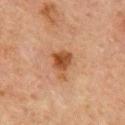| key | value |
|---|---|
| workup | no biopsy performed (imaged during a skin exam) |
| body site | the upper back |
| patient | male, aged around 65 |
| acquisition | 15 mm crop, total-body photography |